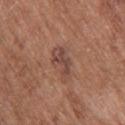Context: Imaged with white-light lighting. A 15 mm close-up extracted from a 3D total-body photography capture. From the upper back. The subject is a male aged 73–77. The total-body-photography lesion software estimated a lesion area of about 7 mm², a shape eccentricity near 0.85, and a symmetry-axis asymmetry near 0.4. The software also gave a within-lesion color-variation index near 4/10. Approximately 4 mm at its widest.